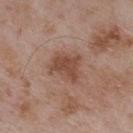Assessment: The lesion was tiled from a total-body skin photograph and was not biopsied. Clinical summary: A 15 mm close-up extracted from a 3D total-body photography capture. The lesion-visualizer software estimated an average lesion color of about L≈48 a*≈20 b*≈28 (CIELAB), a lesion–skin lightness drop of about 9, and a normalized border contrast of about 7.5. It also reported border irregularity of about 4 on a 0–10 scale, a color-variation rating of about 2.5/10, and radial color variation of about 1. A male patient, aged approximately 55. The lesion is on the upper back.